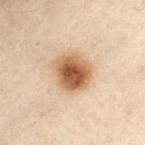{"biopsy_status": "not biopsied; imaged during a skin examination", "patient": {"sex": "female", "age_approx": 55}, "lesion_size": {"long_diameter_mm_approx": 4.5}, "site": "front of the torso", "image": {"source": "total-body photography crop", "field_of_view_mm": 15}}